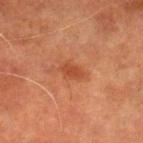follow-up: total-body-photography surveillance lesion; no biopsy.